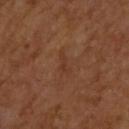<record>
  <biopsy_status>not biopsied; imaged during a skin examination</biopsy_status>
  <image>
    <source>total-body photography crop</source>
    <field_of_view_mm>15</field_of_view_mm>
  </image>
  <patient>
    <sex>male</sex>
    <age_approx>65</age_approx>
  </patient>
  <automated_metrics>
    <cielab_L>34</cielab_L>
    <cielab_a>20</cielab_a>
    <cielab_b>29</cielab_b>
    <vs_skin_darker_L>5.0</vs_skin_darker_L>
    <nevus_likeness_0_100>0</nevus_likeness_0_100>
    <lesion_detection_confidence_0_100>100</lesion_detection_confidence_0_100>
  </automated_metrics>
  <lighting>cross-polarized</lighting>
  <site>upper back</site>
  <lesion_size>
    <long_diameter_mm_approx>3.0</long_diameter_mm_approx>
  </lesion_size>
</record>